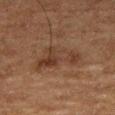The lesion was tiled from a total-body skin photograph and was not biopsied. Cropped from a whole-body photographic skin survey; the tile spans about 15 mm. Captured under cross-polarized illumination. From the right thigh. A male subject, roughly 75 years of age. Automated image analysis of the tile measured an automated nevus-likeness rating near 10 out of 100.